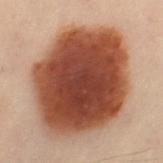Q: Automated lesion metrics?
A: a footprint of about 70 mm², an eccentricity of roughly 0.6, and a shape-asymmetry score of about 0.1 (0 = symmetric)
Q: What are the patient's age and sex?
A: female, about 60 years old
Q: Where on the body is the lesion?
A: the right leg
Q: What is the imaging modality?
A: 15 mm crop, total-body photography
Q: How large is the lesion?
A: ≈11 mm